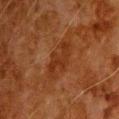biopsy status — imaged on a skin check; not biopsied
acquisition — 15 mm crop, total-body photography
lesion size — ≈4.5 mm
tile lighting — cross-polarized illumination
subject — male, in their 80s
site — the upper back
automated metrics — a footprint of about 9 mm²; a border-irregularity index near 4.5/10, a color-variation rating of about 2.5/10, and radial color variation of about 1